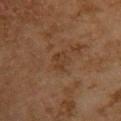Q: Was a biopsy performed?
A: imaged on a skin check; not biopsied
Q: Lesion size?
A: about 2.5 mm
Q: What is the imaging modality?
A: ~15 mm crop, total-body skin-cancer survey
Q: What is the anatomic site?
A: the upper back
Q: Patient demographics?
A: female, in their 60s
Q: Automated lesion metrics?
A: an area of roughly 3.5 mm², an eccentricity of roughly 0.65, and two-axis asymmetry of about 0.35; a nevus-likeness score of about 0/100 and lesion-presence confidence of about 100/100